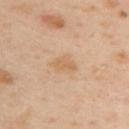| field | value |
|---|---|
| follow-up | imaged on a skin check; not biopsied |
| patient | female, about 40 years old |
| image | 15 mm crop, total-body photography |
| body site | the upper back |
| lighting | cross-polarized |
| size | about 3.5 mm |